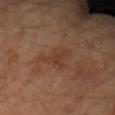Impression:
The lesion was photographed on a routine skin check and not biopsied; there is no pathology result.
Image and clinical context:
A 15 mm close-up tile from a total-body photography series done for melanoma screening. The recorded lesion diameter is about 4 mm. A female patient approximately 60 years of age. The tile uses cross-polarized illumination. Located on the right forearm. The lesion-visualizer software estimated border irregularity of about 4.5 on a 0–10 scale, a color-variation rating of about 2.5/10, and peripheral color asymmetry of about 1. It also reported a detector confidence of about 100 out of 100 that the crop contains a lesion.